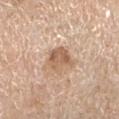| key | value |
|---|---|
| workup | imaged on a skin check; not biopsied |
| image-analysis metrics | an area of roughly 8 mm², a shape eccentricity near 0.45, and a shape-asymmetry score of about 0.3 (0 = symmetric); a mean CIELAB color near L≈60 a*≈19 b*≈32, a lesion–skin lightness drop of about 11, and a lesion-to-skin contrast of about 7.5 (normalized; higher = more distinct); lesion-presence confidence of about 100/100 |
| image source | ~15 mm tile from a whole-body skin photo |
| tile lighting | white-light illumination |
| site | the left lower leg |
| diameter | ≈3.5 mm |
| subject | female, about 70 years old |